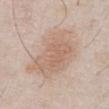{"biopsy_status": "not biopsied; imaged during a skin examination", "site": "front of the torso", "lighting": "white-light", "lesion_size": {"long_diameter_mm_approx": 7.0}, "patient": {"sex": "male", "age_approx": 55}, "image": {"source": "total-body photography crop", "field_of_view_mm": 15}}